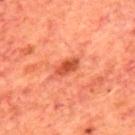{"biopsy_status": "not biopsied; imaged during a skin examination", "automated_metrics": {"cielab_L": 49, "cielab_a": 36, "cielab_b": 39, "vs_skin_darker_L": 12.0, "vs_skin_contrast_norm": 8.0}, "image": {"source": "total-body photography crop", "field_of_view_mm": 15}, "lighting": "cross-polarized", "patient": {"sex": "male", "age_approx": 65}, "lesion_size": {"long_diameter_mm_approx": 3.0}, "site": "upper back"}